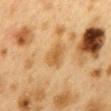No biopsy was performed on this lesion — it was imaged during a full skin examination and was not determined to be concerning. On the back. A 15 mm crop from a total-body photograph taken for skin-cancer surveillance. This is a cross-polarized tile. Automated tile analysis of the lesion measured a lesion area of about 6 mm², an outline eccentricity of about 0.65 (0 = round, 1 = elongated), and two-axis asymmetry of about 0.3. The analysis additionally found about 8 CIELAB-L* units darker than the surrounding skin and a normalized lesion–skin contrast near 6.5. The software also gave an automated nevus-likeness rating near 15 out of 100 and lesion-presence confidence of about 100/100. The patient is a female in their 40s. Measured at roughly 3 mm in maximum diameter.